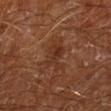The lesion was photographed on a routine skin check and not biopsied; there is no pathology result. A close-up tile cropped from a whole-body skin photograph, about 15 mm across. A male patient aged 63–67. Automated image analysis of the tile measured an area of roughly 6.5 mm², a shape eccentricity near 0.8, and a symmetry-axis asymmetry near 0.5. It also reported a lesion color around L≈31 a*≈22 b*≈28 in CIELAB and a normalized lesion–skin contrast near 6. The software also gave a detector confidence of about 95 out of 100 that the crop contains a lesion. On the left lower leg.